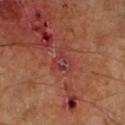Q: Was a biopsy performed?
A: imaged on a skin check; not biopsied
Q: What did automated image analysis measure?
A: a shape eccentricity near 0.5 and two-axis asymmetry of about 0.4; an automated nevus-likeness rating near 0 out of 100 and a lesion-detection confidence of about 100/100
Q: How large is the lesion?
A: ≈2.5 mm
Q: What is the imaging modality?
A: 15 mm crop, total-body photography
Q: Lesion location?
A: the left lower leg
Q: What lighting was used for the tile?
A: cross-polarized illumination
Q: Patient demographics?
A: male, aged 58 to 62